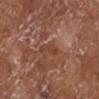Impression:
The lesion was photographed on a routine skin check and not biopsied; there is no pathology result.
Acquisition and patient details:
A 15 mm close-up extracted from a 3D total-body photography capture. A female patient, in their mid- to late 70s. The lesion is on the left lower leg. Longest diameter approximately 3.5 mm.